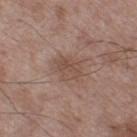Impression:
This lesion was catalogued during total-body skin photography and was not selected for biopsy.
Background:
Imaged with white-light lighting. A male patient in their 50s. Located on the left thigh. Cropped from a whole-body photographic skin survey; the tile spans about 15 mm. About 3.5 mm across. The lesion-visualizer software estimated a footprint of about 6 mm², an eccentricity of roughly 0.75, and a symmetry-axis asymmetry near 0.25. The analysis additionally found a lesion color around L≈49 a*≈17 b*≈25 in CIELAB and roughly 7 lightness units darker than nearby skin.